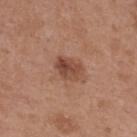Part of a total-body skin-imaging series; this lesion was reviewed on a skin check and was not flagged for biopsy.
Captured under white-light illumination.
A female patient in their 40s.
About 3.5 mm across.
A roughly 15 mm field-of-view crop from a total-body skin photograph.
The lesion is on the back.